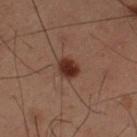Impression: This lesion was catalogued during total-body skin photography and was not selected for biopsy. Background: Located on the left thigh. The subject is a male aged around 60. Automated image analysis of the tile measured a lesion area of about 5 mm² and a shape-asymmetry score of about 0.25 (0 = symmetric). It also reported a lesion color around L≈23 a*≈17 b*≈20 in CIELAB. The analysis additionally found border irregularity of about 2 on a 0–10 scale, a color-variation rating of about 2/10, and peripheral color asymmetry of about 0.5. The recorded lesion diameter is about 2.5 mm. The tile uses cross-polarized illumination. A lesion tile, about 15 mm wide, cut from a 3D total-body photograph.